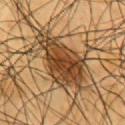biopsy status: imaged on a skin check; not biopsied | diameter: about 8.5 mm | illumination: cross-polarized | image source: total-body-photography crop, ~15 mm field of view | subject: male, aged approximately 60 | site: the front of the torso.